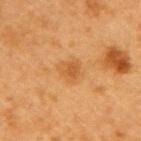Q: Was this lesion biopsied?
A: catalogued during a skin exam; not biopsied
Q: Who is the patient?
A: female, aged 38–42
Q: How large is the lesion?
A: ≈3 mm
Q: How was the tile lit?
A: cross-polarized
Q: What is the imaging modality?
A: total-body-photography crop, ~15 mm field of view
Q: What is the anatomic site?
A: the right upper arm